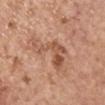| feature | finding |
|---|---|
| biopsy status | imaged on a skin check; not biopsied |
| TBP lesion metrics | a mean CIELAB color near L≈54 a*≈23 b*≈32 and a lesion–skin lightness drop of about 10; a nevus-likeness score of about 0/100 and a lesion-detection confidence of about 100/100 |
| anatomic site | the left upper arm |
| patient | female, approximately 65 years of age |
| tile lighting | white-light |
| acquisition | ~15 mm tile from a whole-body skin photo |
| lesion size | about 6.5 mm |